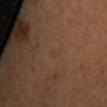| feature | finding |
|---|---|
| workup | no biopsy performed (imaged during a skin exam) |
| imaging modality | 15 mm crop, total-body photography |
| tile lighting | cross-polarized illumination |
| site | the mid back |
| subject | female |